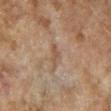Impression: This lesion was catalogued during total-body skin photography and was not selected for biopsy. Background: The lesion is on the leg. This is a cross-polarized tile. This image is a 15 mm lesion crop taken from a total-body photograph. A female patient, about 60 years old. Measured at roughly 2.5 mm in maximum diameter.